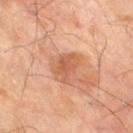This lesion was catalogued during total-body skin photography and was not selected for biopsy. A male patient about 70 years old. Longest diameter approximately 3.5 mm. Captured under cross-polarized illumination. This image is a 15 mm lesion crop taken from a total-body photograph. The lesion is located on the leg.